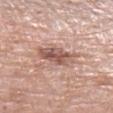patient — male, about 80 years old
lighting — white-light
anatomic site — the leg
image — ~15 mm tile from a whole-body skin photo
size — about 4 mm
TBP lesion metrics — a footprint of about 9.5 mm², an outline eccentricity of about 0.8 (0 = round, 1 = elongated), and a symmetry-axis asymmetry near 0.15; a lesion-to-skin contrast of about 8.5 (normalized; higher = more distinct)